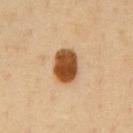<lesion>
  <biopsy_status>not biopsied; imaged during a skin examination</biopsy_status>
  <site>abdomen</site>
  <image>
    <source>total-body photography crop</source>
    <field_of_view_mm>15</field_of_view_mm>
  </image>
  <patient>
    <sex>male</sex>
    <age_approx>50</age_approx>
  </patient>
  <lighting>cross-polarized</lighting>
  <lesion_size>
    <long_diameter_mm_approx>4.0</long_diameter_mm_approx>
  </lesion_size>
</lesion>